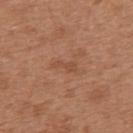follow-up = total-body-photography surveillance lesion; no biopsy
lesion size = ≈3 mm
patient = female, aged 38 to 42
automated metrics = a border-irregularity rating of about 5.5/10, a within-lesion color-variation index near 0/10, and radial color variation of about 0; a nevus-likeness score of about 0/100 and a detector confidence of about 100 out of 100 that the crop contains a lesion
image = ~15 mm crop, total-body skin-cancer survey
location = the upper back
illumination = white-light